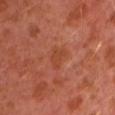Background: The subject is a male approximately 30 years of age. The lesion's longest dimension is about 3 mm. The lesion is located on the arm. Cropped from a total-body skin-imaging series; the visible field is about 15 mm. This is a cross-polarized tile.